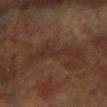| feature | finding |
|---|---|
| follow-up | no biopsy performed (imaged during a skin exam) |
| patient | female, aged around 60 |
| acquisition | total-body-photography crop, ~15 mm field of view |
| illumination | cross-polarized illumination |
| anatomic site | the left forearm |